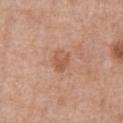The lesion was tiled from a total-body skin photograph and was not biopsied.